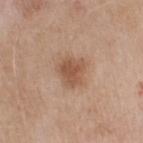{
  "biopsy_status": "not biopsied; imaged during a skin examination",
  "image": {
    "source": "total-body photography crop",
    "field_of_view_mm": 15
  },
  "site": "arm",
  "patient": {
    "sex": "female",
    "age_approx": 55
  }
}